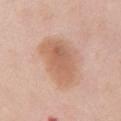This lesion was catalogued during total-body skin photography and was not selected for biopsy. Approximately 7 mm at its widest. A close-up tile cropped from a whole-body skin photograph, about 15 mm across. On the chest. The lesion-visualizer software estimated a lesion area of about 20 mm² and two-axis asymmetry of about 0.2. The software also gave border irregularity of about 2.5 on a 0–10 scale and radial color variation of about 1. A female patient, aged 48–52. Captured under white-light illumination.